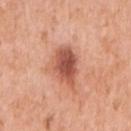No biopsy was performed on this lesion — it was imaged during a full skin examination and was not determined to be concerning. Located on the arm. This image is a 15 mm lesion crop taken from a total-body photograph. The tile uses white-light illumination. A female patient in their 40s. Measured at roughly 5 mm in maximum diameter. Automated tile analysis of the lesion measured a footprint of about 12 mm², an eccentricity of roughly 0.75, and a shape-asymmetry score of about 0.25 (0 = symmetric). And it measured a mean CIELAB color near L≈55 a*≈27 b*≈31, roughly 14 lightness units darker than nearby skin, and a normalized border contrast of about 9. The analysis additionally found border irregularity of about 3 on a 0–10 scale, internal color variation of about 5 on a 0–10 scale, and radial color variation of about 1.5.